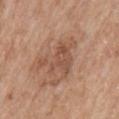biopsy status: imaged on a skin check; not biopsied
site: the mid back
size: about 6 mm
acquisition: total-body-photography crop, ~15 mm field of view
patient: female, in their mid- to late 70s